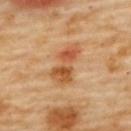Q: Was this lesion biopsied?
A: catalogued during a skin exam; not biopsied
Q: Lesion size?
A: about 5 mm
Q: How was this image acquired?
A: 15 mm crop, total-body photography
Q: Lesion location?
A: the back
Q: What did automated image analysis measure?
A: a footprint of about 9.5 mm², an eccentricity of roughly 0.9, and a shape-asymmetry score of about 0.35 (0 = symmetric); a mean CIELAB color near L≈56 a*≈26 b*≈41, a lesion–skin lightness drop of about 12, and a normalized border contrast of about 8; a border-irregularity index near 4.5/10, a color-variation rating of about 6.5/10, and peripheral color asymmetry of about 2
Q: What are the patient's age and sex?
A: female, in their 60s